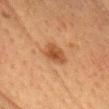{"image": {"source": "total-body photography crop", "field_of_view_mm": 15}, "patient": {"sex": "female", "age_approx": 55}, "site": "head or neck", "lighting": "cross-polarized"}